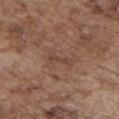<record>
  <biopsy_status>not biopsied; imaged during a skin examination</biopsy_status>
  <image>
    <source>total-body photography crop</source>
    <field_of_view_mm>15</field_of_view_mm>
  </image>
  <patient>
    <sex>male</sex>
    <age_approx>75</age_approx>
  </patient>
  <site>front of the torso</site>
</record>